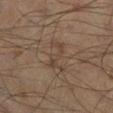A lesion tile, about 15 mm wide, cut from a 3D total-body photograph. Located on the right lower leg. The patient is a male in their mid- to late 50s.